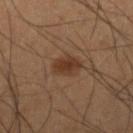The lesion was tiled from a total-body skin photograph and was not biopsied.
The lesion is on the right lower leg.
Automated image analysis of the tile measured a lesion color around L≈35 a*≈19 b*≈28 in CIELAB, roughly 9 lightness units darker than nearby skin, and a normalized border contrast of about 8. It also reported a border-irregularity rating of about 2/10 and internal color variation of about 2.5 on a 0–10 scale. The software also gave lesion-presence confidence of about 100/100.
A close-up tile cropped from a whole-body skin photograph, about 15 mm across.
A male patient, in their 40s.
About 3.5 mm across.
Captured under cross-polarized illumination.